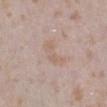| feature | finding |
|---|---|
| workup | no biopsy performed (imaged during a skin exam) |
| anatomic site | the right lower leg |
| lesion diameter | about 3.5 mm |
| TBP lesion metrics | an area of roughly 6 mm², an eccentricity of roughly 0.85, and a symmetry-axis asymmetry near 0.4; about 5 CIELAB-L* units darker than the surrounding skin and a normalized lesion–skin contrast near 4.5; a border-irregularity rating of about 4.5/10, a within-lesion color-variation index near 2.5/10, and peripheral color asymmetry of about 1 |
| patient | female, aged around 25 |
| image source | ~15 mm crop, total-body skin-cancer survey |